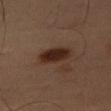Background: From the right thigh. A male patient aged 48 to 52. About 4.5 mm across. The total-body-photography lesion software estimated an area of roughly 8.5 mm², an outline eccentricity of about 0.85 (0 = round, 1 = elongated), and a shape-asymmetry score of about 0.2 (0 = symmetric). And it measured a border-irregularity index near 2/10, a color-variation rating of about 3/10, and radial color variation of about 1. The analysis additionally found a classifier nevus-likeness of about 100/100 and lesion-presence confidence of about 100/100. The tile uses cross-polarized illumination. A 15 mm close-up extracted from a 3D total-body photography capture.